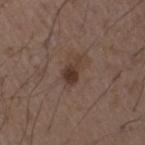The lesion was tiled from a total-body skin photograph and was not biopsied. The total-body-photography lesion software estimated a footprint of about 4.5 mm², an eccentricity of roughly 0.85, and a shape-asymmetry score of about 0.4 (0 = symmetric). The software also gave a lesion color around L≈35 a*≈16 b*≈23 in CIELAB, roughly 9 lightness units darker than nearby skin, and a lesion-to-skin contrast of about 8.5 (normalized; higher = more distinct). Measured at roughly 3.5 mm in maximum diameter. Captured under white-light illumination. A male patient, in their 50s. On the arm. A close-up tile cropped from a whole-body skin photograph, about 15 mm across.